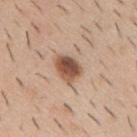Notes:
– biopsy status — no biopsy performed (imaged during a skin exam)
– anatomic site — the mid back
– automated lesion analysis — a lesion color around L≈53 a*≈20 b*≈29 in CIELAB, about 16 CIELAB-L* units darker than the surrounding skin, and a normalized border contrast of about 10.5; a border-irregularity index near 2/10, a color-variation rating of about 6.5/10, and radial color variation of about 2; a nevus-likeness score of about 95/100 and lesion-presence confidence of about 100/100
– diameter — ≈3.5 mm
– acquisition — total-body-photography crop, ~15 mm field of view
– patient — male, about 40 years old
– lighting — white-light illumination